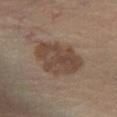follow-up: total-body-photography surveillance lesion; no biopsy
patient: male, aged 58 to 62
automated metrics: a lesion color around L≈41 a*≈14 b*≈24 in CIELAB, a lesion–skin lightness drop of about 9, and a normalized lesion–skin contrast near 7.5; a detector confidence of about 100 out of 100 that the crop contains a lesion
size: ~6 mm (longest diameter)
image source: total-body-photography crop, ~15 mm field of view
lighting: cross-polarized
body site: the left lower leg